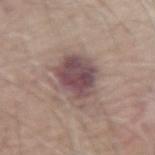Clinical impression: The lesion was photographed on a routine skin check and not biopsied; there is no pathology result. Clinical summary: The tile uses white-light illumination. A male patient approximately 65 years of age. The lesion is located on the left forearm. This image is a 15 mm lesion crop taken from a total-body photograph. The lesion's longest dimension is about 5 mm.